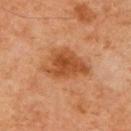workup = no biopsy performed (imaged during a skin exam); patient = male, aged approximately 60; lighting = cross-polarized illumination; imaging modality = 15 mm crop, total-body photography; location = the right upper arm.